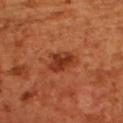<record>
  <patient>
    <sex>female</sex>
    <age_approx>55</age_approx>
  </patient>
  <site>upper back</site>
  <lesion_size>
    <long_diameter_mm_approx>3.5</long_diameter_mm_approx>
  </lesion_size>
  <image>
    <source>total-body photography crop</source>
    <field_of_view_mm>15</field_of_view_mm>
  </image>
  <lighting>cross-polarized</lighting>
</record>